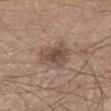Impression: Part of a total-body skin-imaging series; this lesion was reviewed on a skin check and was not flagged for biopsy. Context: A male patient, approximately 45 years of age. Captured under white-light illumination. A lesion tile, about 15 mm wide, cut from a 3D total-body photograph. Located on the right lower leg.